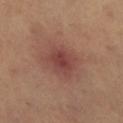Case summary:
- workup: total-body-photography surveillance lesion; no biopsy
- anatomic site: the right lower leg
- imaging modality: 15 mm crop, total-body photography
- subject: female, aged 68 to 72